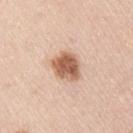| feature | finding |
|---|---|
| lighting | white-light illumination |
| subject | male, about 45 years old |
| body site | the right upper arm |
| size | about 3.5 mm |
| image | ~15 mm crop, total-body skin-cancer survey |
| TBP lesion metrics | a detector confidence of about 100 out of 100 that the crop contains a lesion |